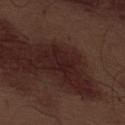Part of a total-body skin-imaging series; this lesion was reviewed on a skin check and was not flagged for biopsy. A male subject, aged 68–72. The tile uses white-light illumination. The lesion's longest dimension is about 3 mm. The lesion is on the abdomen. An algorithmic analysis of the crop reported a footprint of about 3.5 mm², an eccentricity of roughly 0.85, and a symmetry-axis asymmetry near 0.6. The software also gave a lesion color around L≈19 a*≈19 b*≈16 in CIELAB and a normalized lesion–skin contrast near 7.5. The software also gave a color-variation rating of about 0/10 and radial color variation of about 0. It also reported a nevus-likeness score of about 0/100 and a detector confidence of about 70 out of 100 that the crop contains a lesion. A 15 mm close-up extracted from a 3D total-body photography capture.